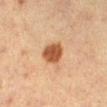Assessment: The lesion was tiled from a total-body skin photograph and was not biopsied. Clinical summary: The subject is a female approximately 55 years of age. Cropped from a whole-body photographic skin survey; the tile spans about 15 mm. Located on the leg.